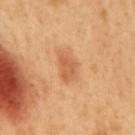No biopsy was performed on this lesion — it was imaged during a full skin examination and was not determined to be concerning.
Imaged with cross-polarized lighting.
The lesion's longest dimension is about 3.5 mm.
The lesion-visualizer software estimated a lesion color around L≈59 a*≈25 b*≈39 in CIELAB and about 9 CIELAB-L* units darker than the surrounding skin. The analysis additionally found a classifier nevus-likeness of about 60/100 and lesion-presence confidence of about 100/100.
A male patient approximately 50 years of age.
On the mid back.
A 15 mm close-up extracted from a 3D total-body photography capture.